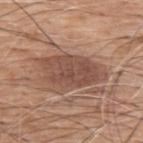<record>
<biopsy_status>not biopsied; imaged during a skin examination</biopsy_status>
<site>upper back</site>
<patient>
  <sex>male</sex>
  <age_approx>60</age_approx>
</patient>
<image>
  <source>total-body photography crop</source>
  <field_of_view_mm>15</field_of_view_mm>
</image>
<automated_metrics>
  <area_mm2_approx>19.0</area_mm2_approx>
  <eccentricity>0.85</eccentricity>
  <shape_asymmetry>0.25</shape_asymmetry>
  <cielab_L>49</cielab_L>
  <cielab_a>20</cielab_a>
  <cielab_b>27</cielab_b>
  <vs_skin_darker_L>11.0</vs_skin_darker_L>
  <vs_skin_contrast_norm>8.0</vs_skin_contrast_norm>
  <lesion_detection_confidence_0_100>100</lesion_detection_confidence_0_100>
</automated_metrics>
<lesion_size>
  <long_diameter_mm_approx>7.0</long_diameter_mm_approx>
</lesion_size>
<lighting>white-light</lighting>
</record>